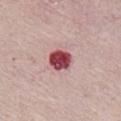  biopsy_status: not biopsied; imaged during a skin examination
  image:
    source: total-body photography crop
    field_of_view_mm: 15
  lighting: white-light
  patient:
    sex: female
    age_approx: 65
  lesion_size:
    long_diameter_mm_approx: 3.0
  automated_metrics:
    area_mm2_approx: 6.5
    shape_asymmetry: 0.2
    vs_skin_darker_L: 22.0
    border_irregularity_0_10: 1.5
    color_variation_0_10: 6.0
    peripheral_color_asymmetry: 2.0
  site: chest